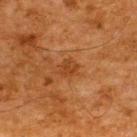Q: Was this lesion biopsied?
A: no biopsy performed (imaged during a skin exam)
Q: Where on the body is the lesion?
A: the upper back
Q: What lighting was used for the tile?
A: cross-polarized illumination
Q: How was this image acquired?
A: 15 mm crop, total-body photography
Q: What are the patient's age and sex?
A: male, approximately 65 years of age